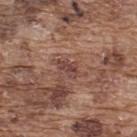Impression: Imaged during a routine full-body skin examination; the lesion was not biopsied and no histopathology is available. Acquisition and patient details: A male subject, aged approximately 75. A 15 mm close-up extracted from a 3D total-body photography capture. This is a white-light tile. The recorded lesion diameter is about 3 mm. On the upper back. Automated image analysis of the tile measured a lesion area of about 3.5 mm², a shape eccentricity near 0.8, and a shape-asymmetry score of about 0.3 (0 = symmetric).